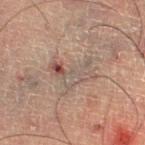Clinical impression:
Part of a total-body skin-imaging series; this lesion was reviewed on a skin check and was not flagged for biopsy.
Background:
This image is a 15 mm lesion crop taken from a total-body photograph. Captured under cross-polarized illumination. The lesion's longest dimension is about 5 mm. A male subject aged 58–62.